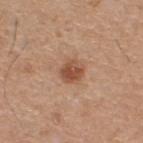Clinical summary:
This is a white-light tile. The subject is a male approximately 60 years of age. Located on the back. Approximately 3 mm at its widest. A 15 mm close-up tile from a total-body photography series done for melanoma screening.